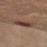<record>
<biopsy_status>not biopsied; imaged during a skin examination</biopsy_status>
<lesion_size>
  <long_diameter_mm_approx>6.5</long_diameter_mm_approx>
</lesion_size>
<lighting>cross-polarized</lighting>
<automated_metrics>
  <cielab_L>40</cielab_L>
  <cielab_a>22</cielab_a>
  <cielab_b>23</cielab_b>
  <vs_skin_darker_L>8.0</vs_skin_darker_L>
</automated_metrics>
<site>right thigh</site>
<patient>
  <sex>female</sex>
  <age_approx>40</age_approx>
</patient>
<image>
  <source>total-body photography crop</source>
  <field_of_view_mm>15</field_of_view_mm>
</image>
</record>